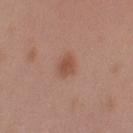biopsy status = imaged on a skin check; not biopsied | subject = male, in their mid- to late 50s | acquisition = 15 mm crop, total-body photography | tile lighting = white-light | site = the left upper arm | automated lesion analysis = a lesion area of about 4 mm², a shape eccentricity near 0.7, and two-axis asymmetry of about 0.25; roughly 9 lightness units darker than nearby skin; border irregularity of about 2 on a 0–10 scale, internal color variation of about 2.5 on a 0–10 scale, and radial color variation of about 1.